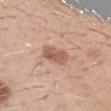Clinical impression:
Imaged during a routine full-body skin examination; the lesion was not biopsied and no histopathology is available.
Image and clinical context:
The patient is a male about 30 years old. This is a white-light tile. The lesion is located on the arm. A region of skin cropped from a whole-body photographic capture, roughly 15 mm wide. The lesion's longest dimension is about 3 mm. An algorithmic analysis of the crop reported a nevus-likeness score of about 75/100 and a detector confidence of about 100 out of 100 that the crop contains a lesion.